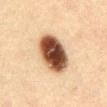{"image": {"source": "total-body photography crop", "field_of_view_mm": 15}, "automated_metrics": {"area_mm2_approx": 18.0, "eccentricity": 0.65, "cielab_L": 39, "cielab_a": 17, "cielab_b": 26, "vs_skin_darker_L": 22.0, "vs_skin_contrast_norm": 16.0, "nevus_likeness_0_100": 100, "lesion_detection_confidence_0_100": 100}, "lesion_size": {"long_diameter_mm_approx": 5.5}, "patient": {"sex": "male", "age_approx": 50}, "site": "abdomen", "lighting": "cross-polarized"}